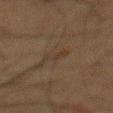Assessment:
Captured during whole-body skin photography for melanoma surveillance; the lesion was not biopsied.
Background:
Located on the mid back. This is a cross-polarized tile. A male subject, aged around 60. The recorded lesion diameter is about 3 mm. A close-up tile cropped from a whole-body skin photograph, about 15 mm across.